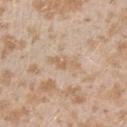{
  "biopsy_status": "not biopsied; imaged during a skin examination",
  "lighting": "white-light",
  "automated_metrics": {
    "vs_skin_darker_L": 7.0,
    "vs_skin_contrast_norm": 5.5,
    "color_variation_0_10": 0.0,
    "peripheral_color_asymmetry": 0.0
  },
  "lesion_size": {
    "long_diameter_mm_approx": 3.5
  },
  "patient": {
    "sex": "female",
    "age_approx": 25
  },
  "image": {
    "source": "total-body photography crop",
    "field_of_view_mm": 15
  },
  "site": "arm"
}